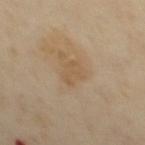This lesion was catalogued during total-body skin photography and was not selected for biopsy. Cropped from a whole-body photographic skin survey; the tile spans about 15 mm. The recorded lesion diameter is about 3 mm. Automated image analysis of the tile measured an area of roughly 4 mm², an eccentricity of roughly 0.8, and two-axis asymmetry of about 0.4. It also reported an average lesion color of about L≈55 a*≈15 b*≈34 (CIELAB), about 6 CIELAB-L* units darker than the surrounding skin, and a lesion-to-skin contrast of about 4.5 (normalized; higher = more distinct). A male patient, about 55 years old. On the mid back.